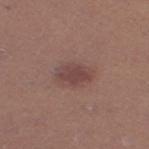Assessment: Part of a total-body skin-imaging series; this lesion was reviewed on a skin check and was not flagged for biopsy. Clinical summary: Longest diameter approximately 3.5 mm. Cropped from a whole-body photographic skin survey; the tile spans about 15 mm. A female subject, in their 40s. Automated image analysis of the tile measured a mean CIELAB color near L≈43 a*≈20 b*≈20, roughly 8 lightness units darker than nearby skin, and a normalized border contrast of about 7. It also reported border irregularity of about 2 on a 0–10 scale, internal color variation of about 2 on a 0–10 scale, and peripheral color asymmetry of about 0.5. It also reported a classifier nevus-likeness of about 35/100. Located on the right thigh.